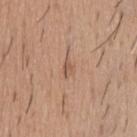No biopsy was performed on this lesion — it was imaged during a full skin examination and was not determined to be concerning. Cropped from a whole-body photographic skin survey; the tile spans about 15 mm. This is a white-light tile. The subject is a male approximately 60 years of age. Automated tile analysis of the lesion measured a footprint of about 2.5 mm², an outline eccentricity of about 0.85 (0 = round, 1 = elongated), and two-axis asymmetry of about 0.3. The analysis additionally found border irregularity of about 3.5 on a 0–10 scale and a color-variation rating of about 1/10. The lesion is located on the upper back.